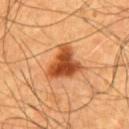{
  "biopsy_status": "not biopsied; imaged during a skin examination",
  "site": "chest",
  "lighting": "cross-polarized",
  "patient": {
    "sex": "male",
    "age_approx": 55
  },
  "image": {
    "source": "total-body photography crop",
    "field_of_view_mm": 15
  }
}